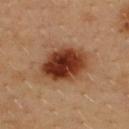- notes: total-body-photography surveillance lesion; no biopsy
- location: the upper back
- patient: male, aged 38–42
- image source: ~15 mm tile from a whole-body skin photo
- lesion size: ~5.5 mm (longest diameter)
- tile lighting: cross-polarized illumination